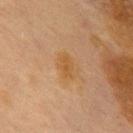Imaged during a routine full-body skin examination; the lesion was not biopsied and no histopathology is available.
On the chest.
Longest diameter approximately 3.5 mm.
The subject is a female approximately 60 years of age.
This is a cross-polarized tile.
A 15 mm close-up tile from a total-body photography series done for melanoma screening.